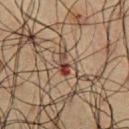Captured during whole-body skin photography for melanoma surveillance; the lesion was not biopsied. Captured under cross-polarized illumination. On the chest. A roughly 15 mm field-of-view crop from a total-body skin photograph. Measured at roughly 3 mm in maximum diameter. A male patient aged 58 to 62. The lesion-visualizer software estimated a footprint of about 3.5 mm², a shape eccentricity near 0.9, and two-axis asymmetry of about 0.3. The software also gave roughly 10 lightness units darker than nearby skin and a normalized border contrast of about 9.5. It also reported peripheral color asymmetry of about 0.5.